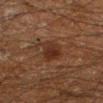Impression:
No biopsy was performed on this lesion — it was imaged during a full skin examination and was not determined to be concerning.
Acquisition and patient details:
Captured under cross-polarized illumination. The subject is a male aged 58 to 62. Measured at roughly 3.5 mm in maximum diameter. A 15 mm close-up extracted from a 3D total-body photography capture. The lesion is on the left lower leg.